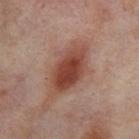Q: Was this lesion biopsied?
A: imaged on a skin check; not biopsied
Q: What is the lesion's diameter?
A: ~7 mm (longest diameter)
Q: Where on the body is the lesion?
A: the right thigh
Q: What is the imaging modality?
A: total-body-photography crop, ~15 mm field of view
Q: Automated lesion metrics?
A: a border-irregularity rating of about 2.5/10, a within-lesion color-variation index near 6/10, and peripheral color asymmetry of about 1.5; an automated nevus-likeness rating near 95 out of 100 and a detector confidence of about 100 out of 100 that the crop contains a lesion
Q: Who is the patient?
A: female, roughly 55 years of age